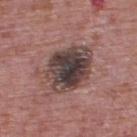No biopsy was performed on this lesion — it was imaged during a full skin examination and was not determined to be concerning. Cropped from a total-body skin-imaging series; the visible field is about 15 mm. The tile uses white-light illumination. The subject is a male approximately 75 years of age. An algorithmic analysis of the crop reported a lesion area of about 21 mm² and a shape eccentricity near 0.55. The software also gave an average lesion color of about L≈40 a*≈15 b*≈17 (CIELAB), roughly 15 lightness units darker than nearby skin, and a lesion-to-skin contrast of about 12 (normalized; higher = more distinct). From the back. The lesion's longest dimension is about 5.5 mm.